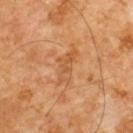  biopsy_status: not biopsied; imaged during a skin examination
  patient:
    sex: male
    age_approx: 60
  image:
    source: total-body photography crop
    field_of_view_mm: 15
  lighting: cross-polarized
  automated_metrics:
    border_irregularity_0_10: 5.5
    color_variation_0_10: 3.0
    peripheral_color_asymmetry: 1.0
  site: front of the torso